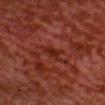Located on the head or neck. A male subject roughly 60 years of age. Longest diameter approximately 2.5 mm. A 15 mm close-up extracted from a 3D total-body photography capture. Automated image analysis of the tile measured an area of roughly 3.5 mm² and a shape eccentricity near 0.75. The software also gave an average lesion color of about L≈19 a*≈23 b*≈22 (CIELAB) and a lesion–skin lightness drop of about 5.